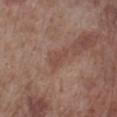{"biopsy_status": "not biopsied; imaged during a skin examination", "lighting": "white-light", "image": {"source": "total-body photography crop", "field_of_view_mm": 15}, "patient": {"sex": "male", "age_approx": 70}, "site": "right lower leg"}